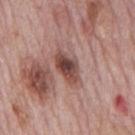Clinical impression:
No biopsy was performed on this lesion — it was imaged during a full skin examination and was not determined to be concerning.
Acquisition and patient details:
A close-up tile cropped from a whole-body skin photograph, about 15 mm across. The lesion is located on the mid back. A male patient about 75 years old. This is a white-light tile. Automated image analysis of the tile measured a lesion area of about 8 mm² and a shape eccentricity near 0.85. The analysis additionally found a mean CIELAB color near L≈46 a*≈21 b*≈23, a lesion–skin lightness drop of about 14, and a normalized border contrast of about 10. And it measured a border-irregularity rating of about 2.5/10 and a peripheral color-asymmetry measure near 1.5. It also reported a nevus-likeness score of about 70/100 and a lesion-detection confidence of about 100/100. About 4.5 mm across.